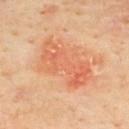follow-up=total-body-photography surveillance lesion; no biopsy | automated metrics=an eccentricity of roughly 0.9 and a symmetry-axis asymmetry near 0.4; a border-irregularity rating of about 6/10 and peripheral color asymmetry of about 2 | tile lighting=cross-polarized | image=~15 mm crop, total-body skin-cancer survey | subject=male, in their mid- to late 60s | anatomic site=the upper back.